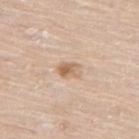Imaged during a routine full-body skin examination; the lesion was not biopsied and no histopathology is available.
From the upper back.
A 15 mm close-up extracted from a 3D total-body photography capture.
A male patient, approximately 75 years of age.
The tile uses white-light illumination.